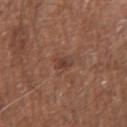Assessment: The lesion was photographed on a routine skin check and not biopsied; there is no pathology result. Acquisition and patient details: On the left forearm. A close-up tile cropped from a whole-body skin photograph, about 15 mm across. An algorithmic analysis of the crop reported a border-irregularity index near 3/10, a color-variation rating of about 3/10, and peripheral color asymmetry of about 1. A male subject, aged around 70.